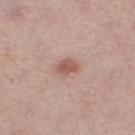Clinical impression:
Captured during whole-body skin photography for melanoma surveillance; the lesion was not biopsied.
Acquisition and patient details:
The tile uses white-light illumination. A 15 mm close-up extracted from a 3D total-body photography capture. From the right thigh. A female subject aged 48 to 52. About 2.5 mm across.